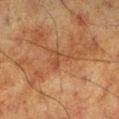Assessment:
The lesion was tiled from a total-body skin photograph and was not biopsied.
Context:
This is a cross-polarized tile. Longest diameter approximately 3 mm. The lesion-visualizer software estimated a lesion area of about 3.5 mm², a shape eccentricity near 0.7, and two-axis asymmetry of about 0.65. The software also gave a border-irregularity rating of about 7.5/10, internal color variation of about 0 on a 0–10 scale, and radial color variation of about 0. The analysis additionally found a classifier nevus-likeness of about 0/100 and a detector confidence of about 95 out of 100 that the crop contains a lesion. A 15 mm close-up extracted from a 3D total-body photography capture. The lesion is located on the left lower leg. The patient is a male aged 58 to 62.